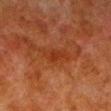workup=imaged on a skin check; not biopsied
patient=male, aged 78 to 82
acquisition=15 mm crop, total-body photography
body site=the left lower leg